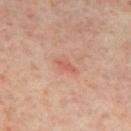biopsy status: total-body-photography surveillance lesion; no biopsy
body site: the mid back
acquisition: 15 mm crop, total-body photography
patient: male, approximately 65 years of age
lighting: cross-polarized illumination
lesion diameter: ≈2.5 mm
TBP lesion metrics: a lesion area of about 2.5 mm², an outline eccentricity of about 0.9 (0 = round, 1 = elongated), and two-axis asymmetry of about 0.4; a lesion color around L≈55 a*≈27 b*≈28 in CIELAB, a lesion–skin lightness drop of about 7, and a normalized border contrast of about 5.5